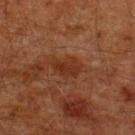| feature | finding |
|---|---|
| notes | imaged on a skin check; not biopsied |
| subject | male, approximately 60 years of age |
| size | about 3 mm |
| image source | ~15 mm tile from a whole-body skin photo |
| lighting | cross-polarized |
| site | the upper back |